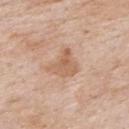Q: Was this lesion biopsied?
A: catalogued during a skin exam; not biopsied
Q: How was this image acquired?
A: 15 mm crop, total-body photography
Q: What is the anatomic site?
A: the upper back
Q: Patient demographics?
A: male, in their mid-80s or older